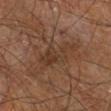This lesion was catalogued during total-body skin photography and was not selected for biopsy. Approximately 6 mm at its widest. The tile uses cross-polarized illumination. The lesion is on the leg. A male patient aged approximately 60. The lesion-visualizer software estimated a border-irregularity index near 6.5/10, a color-variation rating of about 3/10, and radial color variation of about 1. And it measured an automated nevus-likeness rating near 0 out of 100 and a lesion-detection confidence of about 75/100. A roughly 15 mm field-of-view crop from a total-body skin photograph.